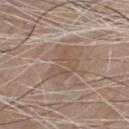Clinical impression:
The lesion was tiled from a total-body skin photograph and was not biopsied.
Clinical summary:
A male subject, aged 78–82. A 15 mm close-up tile from a total-body photography series done for melanoma screening. Located on the front of the torso.